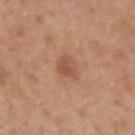Clinical impression: Imaged during a routine full-body skin examination; the lesion was not biopsied and no histopathology is available. Background: From the left forearm. Imaged with white-light lighting. A 15 mm close-up extracted from a 3D total-body photography capture. The lesion's longest dimension is about 2.5 mm. The patient is a female in their 40s.